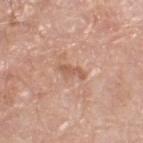The lesion was photographed on a routine skin check and not biopsied; there is no pathology result. About 3 mm across. On the left thigh. A 15 mm close-up extracted from a 3D total-body photography capture. A male patient aged approximately 80. Imaged with white-light lighting.